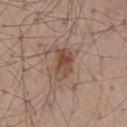{
  "biopsy_status": "not biopsied; imaged during a skin examination",
  "lighting": "white-light",
  "image": {
    "source": "total-body photography crop",
    "field_of_view_mm": 15
  },
  "patient": {
    "sex": "male",
    "age_approx": 55
  },
  "automated_metrics": {
    "cielab_L": 47,
    "cielab_a": 19,
    "cielab_b": 26,
    "vs_skin_darker_L": 10.0,
    "vs_skin_contrast_norm": 8.0
  },
  "site": "mid back"
}